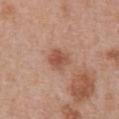Part of a total-body skin-imaging series; this lesion was reviewed on a skin check and was not flagged for biopsy. From the abdomen. Measured at roughly 3 mm in maximum diameter. A male subject about 70 years old. Automated tile analysis of the lesion measured a lesion area of about 5.5 mm², a shape eccentricity near 0.5, and a shape-asymmetry score of about 0.2 (0 = symmetric). The software also gave a mean CIELAB color near L≈52 a*≈24 b*≈30 and a normalized lesion–skin contrast near 7. The software also gave a border-irregularity index near 2/10, a color-variation rating of about 2.5/10, and radial color variation of about 1. A 15 mm close-up tile from a total-body photography series done for melanoma screening.